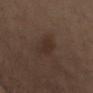Assessment: The lesion was tiled from a total-body skin photograph and was not biopsied. Context: The lesion is on the chest. The lesion-visualizer software estimated a footprint of about 6 mm² and an outline eccentricity of about 0.65 (0 = round, 1 = elongated). The software also gave a classifier nevus-likeness of about 20/100 and lesion-presence confidence of about 100/100. Captured under white-light illumination. A lesion tile, about 15 mm wide, cut from a 3D total-body photograph. The patient is a female roughly 35 years of age.